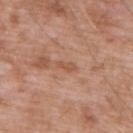follow-up: imaged on a skin check; not biopsied
site: the chest
subject: male, in their 60s
image source: ~15 mm tile from a whole-body skin photo